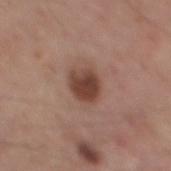Clinical summary: A male patient in their mid- to late 50s. This image is a 15 mm lesion crop taken from a total-body photograph. On the mid back. The total-body-photography lesion software estimated a lesion area of about 8 mm² and a symmetry-axis asymmetry near 0.15. The software also gave a border-irregularity rating of about 1.5/10 and internal color variation of about 4 on a 0–10 scale. It also reported a classifier nevus-likeness of about 95/100. The tile uses white-light illumination. About 3.5 mm across.